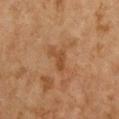follow-up: catalogued during a skin exam; not biopsied
tile lighting: cross-polarized
automated lesion analysis: an area of roughly 3.5 mm², an eccentricity of roughly 0.85, and a shape-asymmetry score of about 0.55 (0 = symmetric); an average lesion color of about L≈41 a*≈20 b*≈33 (CIELAB), about 7 CIELAB-L* units darker than the surrounding skin, and a normalized border contrast of about 6
site: the chest
acquisition: ~15 mm tile from a whole-body skin photo
patient: female, aged 58 to 62